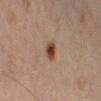Q: Was this lesion biopsied?
A: no biopsy performed (imaged during a skin exam)
Q: Who is the patient?
A: female, roughly 70 years of age
Q: What is the imaging modality?
A: total-body-photography crop, ~15 mm field of view
Q: What is the anatomic site?
A: the right lower leg
Q: What lighting was used for the tile?
A: cross-polarized
Q: How large is the lesion?
A: about 2 mm